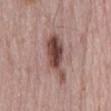biopsy status=catalogued during a skin exam; not biopsied